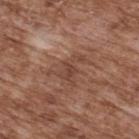Part of a total-body skin-imaging series; this lesion was reviewed on a skin check and was not flagged for biopsy. The subject is a male approximately 75 years of age. A region of skin cropped from a whole-body photographic capture, roughly 15 mm wide. On the upper back.